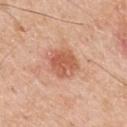Cropped from a total-body skin-imaging series; the visible field is about 15 mm. The recorded lesion diameter is about 3.5 mm. The lesion is located on the mid back. Imaged with white-light lighting. A male subject about 60 years old. An algorithmic analysis of the crop reported an area of roughly 10 mm² and an outline eccentricity of about 0.55 (0 = round, 1 = elongated). The analysis additionally found a border-irregularity rating of about 2/10, a within-lesion color-variation index near 4.5/10, and radial color variation of about 1.5.